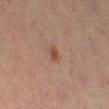Image and clinical context: A female patient approximately 40 years of age. The lesion is on the leg. An algorithmic analysis of the crop reported an area of roughly 3 mm², an eccentricity of roughly 0.6, and a symmetry-axis asymmetry near 0.25. It also reported border irregularity of about 2 on a 0–10 scale, a within-lesion color-variation index near 3.5/10, and a peripheral color-asymmetry measure near 1.5. It also reported an automated nevus-likeness rating near 85 out of 100 and lesion-presence confidence of about 100/100. Approximately 2 mm at its widest. Imaged with cross-polarized lighting. Cropped from a whole-body photographic skin survey; the tile spans about 15 mm.